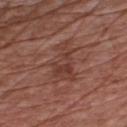{"biopsy_status": "not biopsied; imaged during a skin examination", "site": "chest", "lesion_size": {"long_diameter_mm_approx": 5.0}, "image": {"source": "total-body photography crop", "field_of_view_mm": 15}, "lighting": "white-light", "patient": {"sex": "male", "age_approx": 75}}